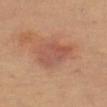The lesion was tiled from a total-body skin photograph and was not biopsied. A close-up tile cropped from a whole-body skin photograph, about 15 mm across. Located on the leg. A female patient aged approximately 70. Measured at roughly 4.5 mm in maximum diameter.